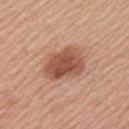No biopsy was performed on this lesion — it was imaged during a full skin examination and was not determined to be concerning. Measured at roughly 4.5 mm in maximum diameter. Imaged with white-light lighting. Automated image analysis of the tile measured an area of roughly 14 mm², an eccentricity of roughly 0.65, and two-axis asymmetry of about 0.15. The analysis additionally found a border-irregularity index near 1.5/10, a within-lesion color-variation index near 4.5/10, and peripheral color asymmetry of about 1.5. Cropped from a total-body skin-imaging series; the visible field is about 15 mm. A female subject, aged approximately 55. The lesion is located on the left upper arm.